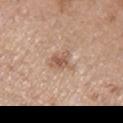Q: Was this lesion biopsied?
A: catalogued during a skin exam; not biopsied
Q: What kind of image is this?
A: ~15 mm crop, total-body skin-cancer survey
Q: Lesion size?
A: about 3 mm
Q: Patient demographics?
A: male, in their mid-50s
Q: Illumination type?
A: white-light illumination
Q: What is the anatomic site?
A: the left upper arm